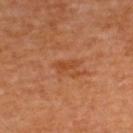Imaged during a routine full-body skin examination; the lesion was not biopsied and no histopathology is available.
The total-body-photography lesion software estimated a lesion color around L≈45 a*≈27 b*≈38 in CIELAB. The analysis additionally found an automated nevus-likeness rating near 0 out of 100 and a detector confidence of about 100 out of 100 that the crop contains a lesion.
Imaged with cross-polarized lighting.
The subject is a female aged 48–52.
The recorded lesion diameter is about 2.5 mm.
From the upper back.
This image is a 15 mm lesion crop taken from a total-body photograph.